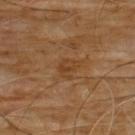Imaged during a routine full-body skin examination; the lesion was not biopsied and no histopathology is available.
On the chest.
Longest diameter approximately 2.5 mm.
A male patient, aged around 60.
A lesion tile, about 15 mm wide, cut from a 3D total-body photograph.
Automated image analysis of the tile measured a lesion area of about 4.5 mm², a shape eccentricity near 0.55, and a symmetry-axis asymmetry near 0.35. The analysis additionally found a mean CIELAB color near L≈39 a*≈20 b*≈34 and a normalized lesion–skin contrast near 5.5. And it measured border irregularity of about 3.5 on a 0–10 scale, a within-lesion color-variation index near 3/10, and radial color variation of about 1.
Captured under cross-polarized illumination.